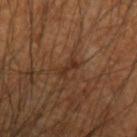<tbp_lesion>
  <biopsy_status>not biopsied; imaged during a skin examination</biopsy_status>
  <image>
    <source>total-body photography crop</source>
    <field_of_view_mm>15</field_of_view_mm>
  </image>
  <lesion_size>
    <long_diameter_mm_approx>2.5</long_diameter_mm_approx>
  </lesion_size>
  <site>arm</site>
  <lighting>cross-polarized</lighting>
  <patient>
    <sex>male</sex>
    <age_approx>60</age_approx>
  </patient>
  <automated_metrics>
    <cielab_L>30</cielab_L>
    <cielab_a>18</cielab_a>
    <cielab_b>27</cielab_b>
    <vs_skin_darker_L>7.0</vs_skin_darker_L>
    <vs_skin_contrast_norm>7.0</vs_skin_contrast_norm>
    <color_variation_0_10>0.0</color_variation_0_10>
    <peripheral_color_asymmetry>0.0</peripheral_color_asymmetry>
  </automated_metrics>
</tbp_lesion>